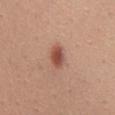Part of a total-body skin-imaging series; this lesion was reviewed on a skin check and was not flagged for biopsy.
The lesion is located on the mid back.
A female subject, aged around 35.
Cropped from a total-body skin-imaging series; the visible field is about 15 mm.
Captured under white-light illumination.
The total-body-photography lesion software estimated a footprint of about 5 mm², an eccentricity of roughly 0.8, and a shape-asymmetry score of about 0.15 (0 = symmetric). And it measured a mean CIELAB color near L≈51 a*≈25 b*≈29, roughly 13 lightness units darker than nearby skin, and a normalized lesion–skin contrast near 9. The analysis additionally found a classifier nevus-likeness of about 100/100 and a lesion-detection confidence of about 100/100.